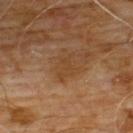No biopsy was performed on this lesion — it was imaged during a full skin examination and was not determined to be concerning. This is a cross-polarized tile. About 3.5 mm across. A male patient aged 58 to 62. A lesion tile, about 15 mm wide, cut from a 3D total-body photograph. The total-body-photography lesion software estimated a lesion color around L≈41 a*≈18 b*≈33 in CIELAB, a lesion–skin lightness drop of about 6, and a lesion-to-skin contrast of about 5.5 (normalized; higher = more distinct). The analysis additionally found border irregularity of about 4.5 on a 0–10 scale, a color-variation rating of about 2/10, and a peripheral color-asymmetry measure near 1. The lesion is on the chest.